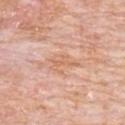follow-up: catalogued during a skin exam; not biopsied
tile lighting: white-light illumination
lesion size: ≈3.5 mm
image: ~15 mm crop, total-body skin-cancer survey
patient: male, aged 78–82
site: the chest